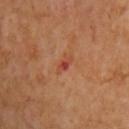Imaged during a routine full-body skin examination; the lesion was not biopsied and no histopathology is available. A roughly 15 mm field-of-view crop from a total-body skin photograph. The subject is a male roughly 60 years of age. The lesion-visualizer software estimated a lesion–skin lightness drop of about 8 and a normalized border contrast of about 6. It also reported a border-irregularity rating of about 3/10, a color-variation rating of about 0/10, and peripheral color asymmetry of about 0. The analysis additionally found a nevus-likeness score of about 0/100 and a lesion-detection confidence of about 100/100. This is a cross-polarized tile. Located on the right upper arm. Measured at roughly 2.5 mm in maximum diameter.